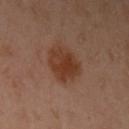No biopsy was performed on this lesion — it was imaged during a full skin examination and was not determined to be concerning.
A female patient about 40 years old.
This is a cross-polarized tile.
A roughly 15 mm field-of-view crop from a total-body skin photograph.
On the left upper arm.
The total-body-photography lesion software estimated an average lesion color of about L≈37 a*≈22 b*≈30 (CIELAB) and a normalized border contrast of about 9. And it measured a nevus-likeness score of about 100/100 and a detector confidence of about 100 out of 100 that the crop contains a lesion.